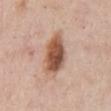| key | value |
|---|---|
| biopsy status | imaged on a skin check; not biopsied |
| automated metrics | a footprint of about 15 mm² and an outline eccentricity of about 0.85 (0 = round, 1 = elongated) |
| tile lighting | white-light |
| acquisition | 15 mm crop, total-body photography |
| patient | male, aged 53 to 57 |
| lesion diameter | ~6 mm (longest diameter) |
| anatomic site | the abdomen |